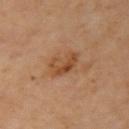Recorded during total-body skin imaging; not selected for excision or biopsy. A female subject aged 58 to 62. On the right upper arm. Cropped from a whole-body photographic skin survey; the tile spans about 15 mm. The tile uses cross-polarized illumination. About 3.5 mm across.